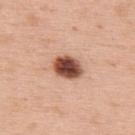{"biopsy_status": "not biopsied; imaged during a skin examination", "site": "upper back", "lighting": "white-light", "lesion_size": {"long_diameter_mm_approx": 3.5}, "image": {"source": "total-body photography crop", "field_of_view_mm": 15}, "patient": {"sex": "male", "age_approx": 60}, "automated_metrics": {"border_irregularity_0_10": 1.5, "color_variation_0_10": 6.0, "peripheral_color_asymmetry": 1.5}}